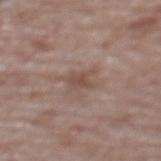Recorded during total-body skin imaging; not selected for excision or biopsy. An algorithmic analysis of the crop reported an area of roughly 3.5 mm² and an eccentricity of roughly 0.75. The software also gave an automated nevus-likeness rating near 0 out of 100 and lesion-presence confidence of about 100/100. A 15 mm crop from a total-body photograph taken for skin-cancer surveillance. Located on the upper back. A female subject, aged approximately 65. This is a white-light tile. Approximately 3 mm at its widest.